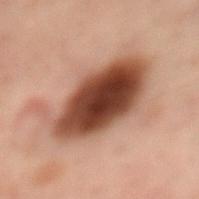  biopsy_status: not biopsied; imaged during a skin examination
  image:
    source: total-body photography crop
    field_of_view_mm: 15
  site: mid back
  patient:
    sex: female
    age_approx: 55